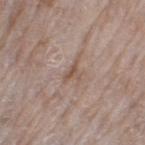Recorded during total-body skin imaging; not selected for excision or biopsy.
A female subject in their mid-70s.
From the left thigh.
A close-up tile cropped from a whole-body skin photograph, about 15 mm across.